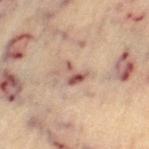<case>
  <lesion_size>
    <long_diameter_mm_approx>3.0</long_diameter_mm_approx>
  </lesion_size>
  <patient>
    <sex>female</sex>
    <age_approx>65</age_approx>
  </patient>
  <image>
    <source>total-body photography crop</source>
    <field_of_view_mm>15</field_of_view_mm>
  </image>
  <site>left thigh</site>
  <automated_metrics>
    <area_mm2_approx>4.0</area_mm2_approx>
    <eccentricity>0.65</eccentricity>
    <shape_asymmetry>0.6</shape_asymmetry>
    <cielab_L>53</cielab_L>
    <cielab_a>21</cielab_a>
    <cielab_b>24</cielab_b>
    <vs_skin_contrast_norm>8.5</vs_skin_contrast_norm>
    <lesion_detection_confidence_0_100>100</lesion_detection_confidence_0_100>
  </automated_metrics>
  <lighting>cross-polarized</lighting>
</case>